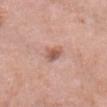Captured during whole-body skin photography for melanoma surveillance; the lesion was not biopsied. Captured under white-light illumination. A 15 mm crop from a total-body photograph taken for skin-cancer surveillance. On the head or neck. A female patient in their 60s. The lesion's longest dimension is about 2.5 mm.